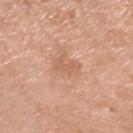Part of a total-body skin-imaging series; this lesion was reviewed on a skin check and was not flagged for biopsy. The recorded lesion diameter is about 3 mm. The lesion is on the left lower leg. A roughly 15 mm field-of-view crop from a total-body skin photograph. The subject is a male about 80 years old.